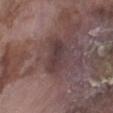Captured during whole-body skin photography for melanoma surveillance; the lesion was not biopsied.
A 15 mm crop from a total-body photograph taken for skin-cancer surveillance.
The lesion is on the left lower leg.
The recorded lesion diameter is about 4.5 mm.
The subject is a male approximately 75 years of age.